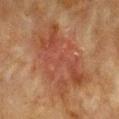This lesion was catalogued during total-body skin photography and was not selected for biopsy.
A female patient, aged approximately 55.
Captured under cross-polarized illumination.
A lesion tile, about 15 mm wide, cut from a 3D total-body photograph.
Automated tile analysis of the lesion measured an average lesion color of about L≈39 a*≈21 b*≈28 (CIELAB) and a lesion-to-skin contrast of about 6 (normalized; higher = more distinct). And it measured an automated nevus-likeness rating near 0 out of 100 and a lesion-detection confidence of about 100/100.
On the chest.
Approximately 9.5 mm at its widest.